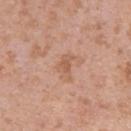This lesion was catalogued during total-body skin photography and was not selected for biopsy. About 2.5 mm across. Cropped from a total-body skin-imaging series; the visible field is about 15 mm. A male subject, roughly 40 years of age. The lesion-visualizer software estimated a lesion color around L≈58 a*≈22 b*≈32 in CIELAB, about 7 CIELAB-L* units darker than the surrounding skin, and a normalized border contrast of about 5.5. And it measured an automated nevus-likeness rating near 0 out of 100 and a lesion-detection confidence of about 100/100. This is a white-light tile. Located on the left upper arm.